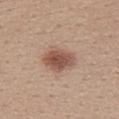Assessment: No biopsy was performed on this lesion — it was imaged during a full skin examination and was not determined to be concerning. Background: The lesion is located on the back. A 15 mm close-up tile from a total-body photography series done for melanoma screening. The subject is a female aged approximately 30.